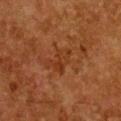Case summary:
* notes · catalogued during a skin exam; not biopsied
* site · the front of the torso
* patient · female, aged approximately 50
* acquisition · 15 mm crop, total-body photography